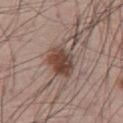No biopsy was performed on this lesion — it was imaged during a full skin examination and was not determined to be concerning. On the chest. A close-up tile cropped from a whole-body skin photograph, about 15 mm across. A male patient aged approximately 55. Imaged with white-light lighting.